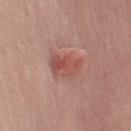Impression:
The lesion was tiled from a total-body skin photograph and was not biopsied.
Background:
The subject is a male in their mid- to late 60s. The tile uses white-light illumination. The lesion is located on the arm. Cropped from a total-body skin-imaging series; the visible field is about 15 mm. The total-body-photography lesion software estimated a footprint of about 7 mm² and two-axis asymmetry of about 0.35. And it measured an automated nevus-likeness rating near 45 out of 100.